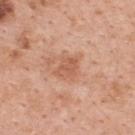Background:
A 15 mm crop from a total-body photograph taken for skin-cancer surveillance. The lesion-visualizer software estimated an eccentricity of roughly 0.6. The lesion is located on the upper back. The tile uses white-light illumination. A female subject, about 50 years old. The lesion's longest dimension is about 3 mm.